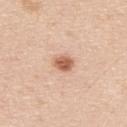Clinical summary:
The tile uses white-light illumination. The total-body-photography lesion software estimated a lesion area of about 4 mm², an eccentricity of roughly 0.65, and a shape-asymmetry score of about 0.15 (0 = symmetric). And it measured a border-irregularity rating of about 1/10, a color-variation rating of about 2/10, and radial color variation of about 0.5. The analysis additionally found a nevus-likeness score of about 95/100 and a lesion-detection confidence of about 100/100. A 15 mm close-up extracted from a 3D total-body photography capture. From the upper back. Approximately 2.5 mm at its widest. A male subject aged approximately 45.